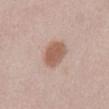biopsy_status: not biopsied; imaged during a skin examination
image:
  source: total-body photography crop
  field_of_view_mm: 15
lesion_size:
  long_diameter_mm_approx: 3.5
lighting: white-light
patient:
  sex: female
  age_approx: 40
site: abdomen